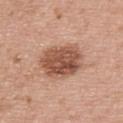Clinical summary: A roughly 15 mm field-of-view crop from a total-body skin photograph. A male patient about 65 years old. From the upper back.